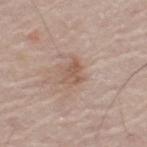Findings:
– biopsy status: catalogued during a skin exam; not biopsied
– subject: male, aged approximately 70
– body site: the right leg
– tile lighting: white-light
– size: ≈3 mm
– image: total-body-photography crop, ~15 mm field of view